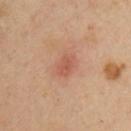follow-up: catalogued during a skin exam; not biopsied | image: 15 mm crop, total-body photography | patient: male, aged approximately 40 | illumination: cross-polarized illumination | site: the front of the torso | lesion diameter: ~3 mm (longest diameter) | TBP lesion metrics: an area of roughly 4 mm² and a symmetry-axis asymmetry near 0.3; about 8 CIELAB-L* units darker than the surrounding skin; border irregularity of about 3 on a 0–10 scale, internal color variation of about 1.5 on a 0–10 scale, and a peripheral color-asymmetry measure near 0.5; an automated nevus-likeness rating near 5 out of 100 and a lesion-detection confidence of about 100/100.